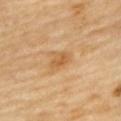The total-body-photography lesion software estimated an area of roughly 4.5 mm², a shape eccentricity near 0.7, and two-axis asymmetry of about 0.3.
About 2.5 mm across.
The patient is a female aged around 60.
On the upper back.
A lesion tile, about 15 mm wide, cut from a 3D total-body photograph.